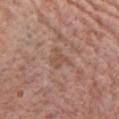The lesion was tiled from a total-body skin photograph and was not biopsied.
A close-up tile cropped from a whole-body skin photograph, about 15 mm across.
From the chest.
The subject is a female in their mid- to late 60s.
About 3 mm across.
Captured under white-light illumination.